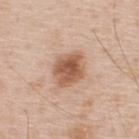workup: imaged on a skin check; not biopsied
location: the upper back
subject: male, in their 50s
diameter: ≈4 mm
image source: 15 mm crop, total-body photography
automated lesion analysis: a footprint of about 10 mm², a shape eccentricity near 0.65, and a shape-asymmetry score of about 0.2 (0 = symmetric); peripheral color asymmetry of about 1.5